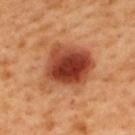Impression:
Recorded during total-body skin imaging; not selected for excision or biopsy.
Clinical summary:
Longest diameter approximately 6.5 mm. A 15 mm close-up extracted from a 3D total-body photography capture. Located on the upper back. A male patient in their 50s. Captured under cross-polarized illumination. Automated image analysis of the tile measured roughly 16 lightness units darker than nearby skin and a lesion-to-skin contrast of about 11.5 (normalized; higher = more distinct). It also reported a border-irregularity rating of about 3/10 and a peripheral color-asymmetry measure near 2.5. And it measured a classifier nevus-likeness of about 100/100 and a lesion-detection confidence of about 100/100.